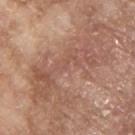Notes:
– subject — male, about 65 years old
– lesion size — ≈10 mm
– image — ~15 mm tile from a whole-body skin photo
– illumination — white-light
– location — the back
– automated metrics — a footprint of about 26 mm², an eccentricity of roughly 0.95, and a symmetry-axis asymmetry near 0.45; a border-irregularity rating of about 8.5/10, a color-variation rating of about 4.5/10, and radial color variation of about 1.5